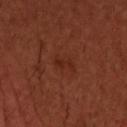Part of a total-body skin-imaging series; this lesion was reviewed on a skin check and was not flagged for biopsy.
A male patient, in their 40s.
The lesion is on the head or neck.
A 15 mm close-up extracted from a 3D total-body photography capture.